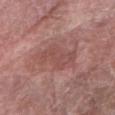notes: no biopsy performed (imaged during a skin exam) | TBP lesion metrics: a footprint of about 12 mm², an outline eccentricity of about 0.8 (0 = round, 1 = elongated), and two-axis asymmetry of about 0.3; border irregularity of about 4 on a 0–10 scale, a within-lesion color-variation index near 2/10, and a peripheral color-asymmetry measure near 1; an automated nevus-likeness rating near 0 out of 100 and a lesion-detection confidence of about 100/100 | patient: male, in their mid-70s | illumination: white-light illumination | acquisition: ~15 mm tile from a whole-body skin photo | anatomic site: the right forearm | size: about 5 mm.